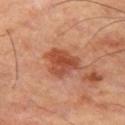Q: Is there a histopathology result?
A: catalogued during a skin exam; not biopsied
Q: Lesion location?
A: the left thigh
Q: What is the lesion's diameter?
A: ≈4.5 mm
Q: What is the imaging modality?
A: ~15 mm crop, total-body skin-cancer survey
Q: What lighting was used for the tile?
A: cross-polarized
Q: What are the patient's age and sex?
A: in their mid-60s